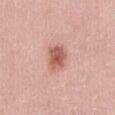The lesion was photographed on a routine skin check and not biopsied; there is no pathology result.
The subject is a female in their mid-40s.
This is a white-light tile.
Located on the abdomen.
This image is a 15 mm lesion crop taken from a total-body photograph.
Automated image analysis of the tile measured a lesion area of about 8.5 mm², an eccentricity of roughly 0.65, and two-axis asymmetry of about 0.2. The analysis additionally found a mean CIELAB color near L≈59 a*≈24 b*≈28, a lesion–skin lightness drop of about 12, and a normalized lesion–skin contrast near 8. The software also gave border irregularity of about 2 on a 0–10 scale, a color-variation rating of about 4/10, and a peripheral color-asymmetry measure near 1. The analysis additionally found a nevus-likeness score of about 85/100 and a lesion-detection confidence of about 100/100.